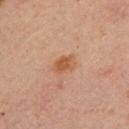No biopsy was performed on this lesion — it was imaged during a full skin examination and was not determined to be concerning. From the left upper arm. The patient is a male aged approximately 30. A roughly 15 mm field-of-view crop from a total-body skin photograph.